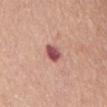  biopsy_status: not biopsied; imaged during a skin examination
  patient:
    sex: female
    age_approx: 55
  site: chest
  image:
    source: total-body photography crop
    field_of_view_mm: 15
  automated_metrics:
    area_mm2_approx: 4.0
    eccentricity: 0.65
    shape_asymmetry: 0.2
    cielab_L: 52
    cielab_a: 29
    cielab_b: 21
    vs_skin_darker_L: 16.0
    border_irregularity_0_10: 2.0
    color_variation_0_10: 4.0
    peripheral_color_asymmetry: 1.5
  lighting: white-light